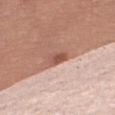The lesion was tiled from a total-body skin photograph and was not biopsied. A female patient, in their mid- to late 60s. Located on the right thigh. The lesion's longest dimension is about 3 mm. A 15 mm crop from a total-body photograph taken for skin-cancer surveillance. Imaged with white-light lighting.